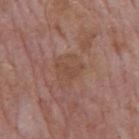Recorded during total-body skin imaging; not selected for excision or biopsy.
The lesion's longest dimension is about 3 mm.
The lesion is on the mid back.
Imaged with white-light lighting.
Cropped from a whole-body photographic skin survey; the tile spans about 15 mm.
A male subject aged around 75.